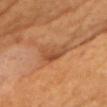Notes:
- workup: no biopsy performed (imaged during a skin exam)
- tile lighting: cross-polarized illumination
- location: the chest
- acquisition: ~15 mm crop, total-body skin-cancer survey
- automated lesion analysis: an eccentricity of roughly 0.9 and two-axis asymmetry of about 0.5; a lesion color around L≈47 a*≈24 b*≈35 in CIELAB, roughly 9 lightness units darker than nearby skin, and a normalized lesion–skin contrast near 6.5; an automated nevus-likeness rating near 10 out of 100 and lesion-presence confidence of about 85/100
- subject: female, aged approximately 70